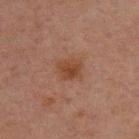Q: Is there a histopathology result?
A: total-body-photography surveillance lesion; no biopsy
Q: Patient demographics?
A: male, in their 30s
Q: What kind of image is this?
A: total-body-photography crop, ~15 mm field of view
Q: Lesion location?
A: the upper back
Q: Automated lesion metrics?
A: a footprint of about 6.5 mm² and a shape eccentricity near 0.55; a lesion color around L≈36 a*≈19 b*≈26 in CIELAB, roughly 7 lightness units darker than nearby skin, and a normalized border contrast of about 7; an automated nevus-likeness rating near 45 out of 100
Q: Illumination type?
A: cross-polarized